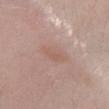- notes — imaged on a skin check; not biopsied
- imaging modality — total-body-photography crop, ~15 mm field of view
- site — the leg
- TBP lesion metrics — two-axis asymmetry of about 0.2; an average lesion color of about L≈58 a*≈18 b*≈26 (CIELAB), about 5 CIELAB-L* units darker than the surrounding skin, and a normalized lesion–skin contrast near 4.5; an automated nevus-likeness rating near 0 out of 100 and a lesion-detection confidence of about 100/100
- size — ~4 mm (longest diameter)
- subject — female, aged 58 to 62